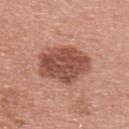Part of a total-body skin-imaging series; this lesion was reviewed on a skin check and was not flagged for biopsy. The lesion is on the upper back. A female subject approximately 40 years of age. Approximately 6 mm at its widest. A 15 mm close-up extracted from a 3D total-body photography capture.